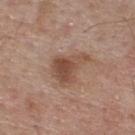The lesion was photographed on a routine skin check and not biopsied; there is no pathology result. On the upper back. A male subject roughly 55 years of age. A roughly 15 mm field-of-view crop from a total-body skin photograph.